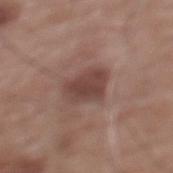Impression:
Part of a total-body skin-imaging series; this lesion was reviewed on a skin check and was not flagged for biopsy.
Image and clinical context:
The lesion is on the mid back. A roughly 15 mm field-of-view crop from a total-body skin photograph. Approximately 4 mm at its widest. A male subject about 60 years old. Captured under white-light illumination.